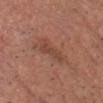| field | value |
|---|---|
| acquisition | 15 mm crop, total-body photography |
| lesion size | ≈3.5 mm |
| patient | male, about 60 years old |
| body site | the head or neck |